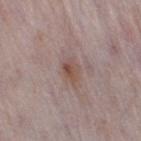biopsy_status: not biopsied; imaged during a skin examination
image:
  source: total-body photography crop
  field_of_view_mm: 15
site: right thigh
patient:
  sex: female
  age_approx: 30
lesion_size:
  long_diameter_mm_approx: 3.0